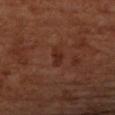  biopsy_status: not biopsied; imaged during a skin examination
  automated_metrics:
    cielab_L: 27
    cielab_a: 22
    cielab_b: 26
    vs_skin_darker_L: 6.0
    vs_skin_contrast_norm: 6.5
    border_irregularity_0_10: 3.0
    color_variation_0_10: 1.0
    peripheral_color_asymmetry: 0.5
    nevus_likeness_0_100: 0
  image:
    source: total-body photography crop
    field_of_view_mm: 15
  lighting: cross-polarized
  patient:
    sex: female
    age_approx: 65
  lesion_size:
    long_diameter_mm_approx: 3.0
  site: left forearm